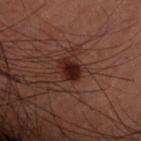Notes:
• lighting: cross-polarized illumination
• image-analysis metrics: a footprint of about 4.5 mm², a shape eccentricity near 0.65, and a shape-asymmetry score of about 0.2 (0 = symmetric); a nevus-likeness score of about 95/100 and lesion-presence confidence of about 100/100
• size: ≈2.5 mm
• patient: male, aged 58 to 62
• location: the right forearm
• image source: total-body-photography crop, ~15 mm field of view